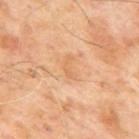Captured during whole-body skin photography for melanoma surveillance; the lesion was not biopsied. The recorded lesion diameter is about 2.5 mm. Imaged with cross-polarized lighting. A roughly 15 mm field-of-view crop from a total-body skin photograph. A male subject, aged 63 to 67. On the mid back.